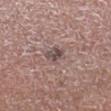Q: Was a biopsy performed?
A: catalogued during a skin exam; not biopsied
Q: Patient demographics?
A: male, in their mid- to late 50s
Q: Lesion location?
A: the right lower leg
Q: How was the tile lit?
A: white-light illumination
Q: What kind of image is this?
A: ~15 mm tile from a whole-body skin photo